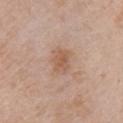Q: Is there a histopathology result?
A: imaged on a skin check; not biopsied
Q: Illumination type?
A: white-light illumination
Q: How was this image acquired?
A: total-body-photography crop, ~15 mm field of view
Q: Patient demographics?
A: female, in their 70s
Q: How large is the lesion?
A: about 3 mm
Q: Where on the body is the lesion?
A: the chest